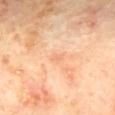Case summary:
* follow-up · imaged on a skin check; not biopsied
* lighting · cross-polarized
* lesion diameter · ≈2 mm
* subject · male, roughly 65 years of age
* image source · total-body-photography crop, ~15 mm field of view
* anatomic site · the mid back